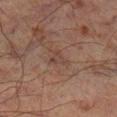Q: Was a biopsy performed?
A: no biopsy performed (imaged during a skin exam)
Q: Lesion size?
A: ≈2.5 mm
Q: Where on the body is the lesion?
A: the left lower leg
Q: What lighting was used for the tile?
A: cross-polarized
Q: What are the patient's age and sex?
A: male, aged 63–67
Q: How was this image acquired?
A: ~15 mm tile from a whole-body skin photo